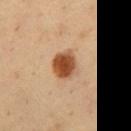follow-up: catalogued during a skin exam; not biopsied | image source: 15 mm crop, total-body photography | size: ≈3.5 mm | patient: male, aged approximately 50 | location: the chest | lighting: cross-polarized.